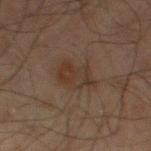Imaged during a routine full-body skin examination; the lesion was not biopsied and no histopathology is available. Located on the left thigh. The lesion-visualizer software estimated a border-irregularity rating of about 4/10, a within-lesion color-variation index near 4/10, and peripheral color asymmetry of about 1. The software also gave lesion-presence confidence of about 100/100. A 15 mm close-up tile from a total-body photography series done for melanoma screening. Measured at roughly 4 mm in maximum diameter. This is a cross-polarized tile. The subject is a male aged approximately 70.